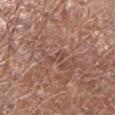A female subject, aged approximately 80. Approximately 2.5 mm at its widest. This is a white-light tile. On the left lower leg. An algorithmic analysis of the crop reported a lesion area of about 2.5 mm² and a symmetry-axis asymmetry near 0.35. The analysis additionally found a border-irregularity rating of about 4/10, a within-lesion color-variation index near 0/10, and radial color variation of about 0. A close-up tile cropped from a whole-body skin photograph, about 15 mm across.